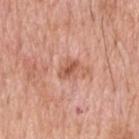Recorded during total-body skin imaging; not selected for excision or biopsy.
A 15 mm crop from a total-body photograph taken for skin-cancer surveillance.
The lesion's longest dimension is about 2.5 mm.
Located on the upper back.
The patient is a male aged approximately 70.
The tile uses white-light illumination.